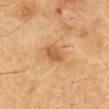The lesion was tiled from a total-body skin photograph and was not biopsied. This is a cross-polarized tile. The patient is a male aged around 50. On the abdomen. The lesion's longest dimension is about 3 mm. A 15 mm close-up extracted from a 3D total-body photography capture.